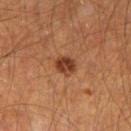Assessment:
The lesion was tiled from a total-body skin photograph and was not biopsied.
Context:
The lesion is located on the left thigh. Cropped from a whole-body photographic skin survey; the tile spans about 15 mm. A male patient, roughly 65 years of age. Approximately 2.5 mm at its widest.